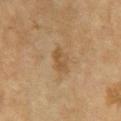notes: total-body-photography surveillance lesion; no biopsy | TBP lesion metrics: a lesion color around L≈46 a*≈15 b*≈33 in CIELAB and a lesion-to-skin contrast of about 6 (normalized; higher = more distinct); a detector confidence of about 100 out of 100 that the crop contains a lesion | body site: the chest | image: ~15 mm tile from a whole-body skin photo | size: ~3.5 mm (longest diameter) | patient: female, approximately 60 years of age | tile lighting: cross-polarized illumination.